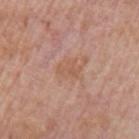notes: no biopsy performed (imaged during a skin exam)
image source: ~15 mm tile from a whole-body skin photo
subject: male, aged around 75
site: the left upper arm